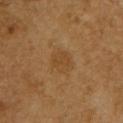notes: catalogued during a skin exam; not biopsied | image: ~15 mm tile from a whole-body skin photo | tile lighting: cross-polarized | patient: male, in their mid-50s | size: ≈3.5 mm | anatomic site: the chest | image-analysis metrics: a border-irregularity rating of about 2/10 and a peripheral color-asymmetry measure near 1; lesion-presence confidence of about 100/100.